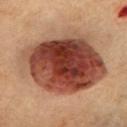biopsy status — no biopsy performed (imaged during a skin exam)
image — 15 mm crop, total-body photography
lighting — cross-polarized illumination
patient — female, aged approximately 60
TBP lesion metrics — a mean CIELAB color near L≈38 a*≈23 b*≈26, roughly 18 lightness units darker than nearby skin, and a lesion-to-skin contrast of about 14 (normalized; higher = more distinct); a classifier nevus-likeness of about 100/100 and a detector confidence of about 100 out of 100 that the crop contains a lesion
body site — the chest